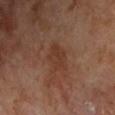Findings:
– workup · no biopsy performed (imaged during a skin exam)
– lesion diameter · ~4 mm (longest diameter)
– patient · male, approximately 70 years of age
– imaging modality · ~15 mm crop, total-body skin-cancer survey
– anatomic site · the chest
– lighting · cross-polarized
– automated metrics · an area of roughly 7.5 mm², an eccentricity of roughly 0.8, and two-axis asymmetry of about 0.3; a within-lesion color-variation index near 2/10 and peripheral color asymmetry of about 0.5; an automated nevus-likeness rating near 0 out of 100